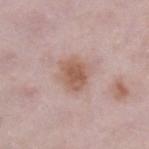Part of a total-body skin-imaging series; this lesion was reviewed on a skin check and was not flagged for biopsy. The lesion is located on the right lower leg. A roughly 15 mm field-of-view crop from a total-body skin photograph. The patient is a female roughly 30 years of age. Approximately 3.5 mm at its widest.